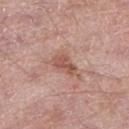Imaged during a routine full-body skin examination; the lesion was not biopsied and no histopathology is available. On the left thigh. A close-up tile cropped from a whole-body skin photograph, about 15 mm across. Automated image analysis of the tile measured a mean CIELAB color near L≈54 a*≈22 b*≈27, roughly 10 lightness units darker than nearby skin, and a normalized lesion–skin contrast near 7. It also reported border irregularity of about 4 on a 0–10 scale, a within-lesion color-variation index near 3.5/10, and radial color variation of about 1.5. A male subject, in their mid-60s. The recorded lesion diameter is about 3.5 mm.